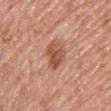biopsy status — imaged on a skin check; not biopsied
lesion size — about 4 mm
tile lighting — white-light
location — the front of the torso
image-analysis metrics — a symmetry-axis asymmetry near 0.25; a lesion color around L≈53 a*≈25 b*≈32 in CIELAB, a lesion–skin lightness drop of about 11, and a normalized lesion–skin contrast near 8; a border-irregularity rating of about 2.5/10, a within-lesion color-variation index near 4/10, and peripheral color asymmetry of about 1.5
imaging modality — 15 mm crop, total-body photography
subject — female, in their 50s